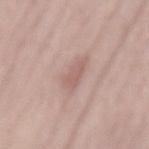Assessment: Part of a total-body skin-imaging series; this lesion was reviewed on a skin check and was not flagged for biopsy. Acquisition and patient details: Measured at roughly 4.5 mm in maximum diameter. A 15 mm close-up tile from a total-body photography series done for melanoma screening. From the mid back. The total-body-photography lesion software estimated a footprint of about 5.5 mm², a shape eccentricity near 0.95, and two-axis asymmetry of about 0.35. It also reported a mean CIELAB color near L≈60 a*≈19 b*≈23, a lesion–skin lightness drop of about 8, and a lesion-to-skin contrast of about 5.5 (normalized; higher = more distinct). The analysis additionally found a classifier nevus-likeness of about 0/100 and a lesion-detection confidence of about 90/100. A male patient, aged approximately 60.